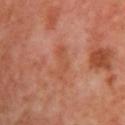A roughly 15 mm field-of-view crop from a total-body skin photograph.
On the right upper arm.
Automated tile analysis of the lesion measured a lesion area of about 5.5 mm² and an eccentricity of roughly 0.9. The analysis additionally found a border-irregularity rating of about 5/10, internal color variation of about 1.5 on a 0–10 scale, and radial color variation of about 0.5.
A female patient, approximately 50 years of age.